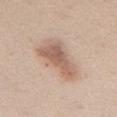Q: Is there a histopathology result?
A: no biopsy performed (imaged during a skin exam)
Q: What is the imaging modality?
A: ~15 mm tile from a whole-body skin photo
Q: What lighting was used for the tile?
A: white-light
Q: What is the anatomic site?
A: the mid back
Q: Lesion size?
A: about 6.5 mm
Q: Who is the patient?
A: female, aged around 40